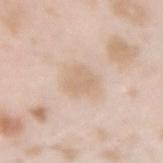{"biopsy_status": "not biopsied; imaged during a skin examination", "patient": {"sex": "female", "age_approx": 25}, "image": {"source": "total-body photography crop", "field_of_view_mm": 15}, "site": "right forearm"}